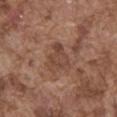- follow-up — catalogued during a skin exam; not biopsied
- location — the front of the torso
- patient — male, in their mid-70s
- image — ~15 mm crop, total-body skin-cancer survey
- lesion size — ~3.5 mm (longest diameter)
- lighting — white-light